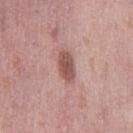imaging modality: ~15 mm crop, total-body skin-cancer survey
diameter: ≈4 mm
body site: the left thigh
patient: female, about 40 years old
illumination: white-light illumination
automated lesion analysis: a footprint of about 6.5 mm² and an eccentricity of roughly 0.85; a border-irregularity rating of about 1.5/10 and a within-lesion color-variation index near 3/10; an automated nevus-likeness rating near 85 out of 100